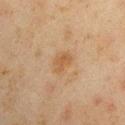The lesion was photographed on a routine skin check and not biopsied; there is no pathology result. Automated image analysis of the tile measured a footprint of about 4.5 mm² and an eccentricity of roughly 0.75. It also reported a lesion color around L≈45 a*≈16 b*≈31 in CIELAB, about 6 CIELAB-L* units darker than the surrounding skin, and a normalized lesion–skin contrast near 6.5. The analysis additionally found a border-irregularity index near 3.5/10, a within-lesion color-variation index near 2/10, and radial color variation of about 1. A 15 mm crop from a total-body photograph taken for skin-cancer surveillance. A male subject, roughly 45 years of age. From the right upper arm. Imaged with cross-polarized lighting. The recorded lesion diameter is about 3 mm.